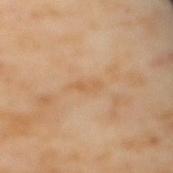notes: no biopsy performed (imaged during a skin exam) | lesion diameter: ~3 mm (longest diameter) | automated lesion analysis: a footprint of about 2.5 mm² and an eccentricity of roughly 0.95; a mean CIELAB color near L≈61 a*≈19 b*≈39, a lesion–skin lightness drop of about 6, and a normalized lesion–skin contrast near 5; a classifier nevus-likeness of about 0/100 and a detector confidence of about 100 out of 100 that the crop contains a lesion | anatomic site: the back | lighting: cross-polarized | subject: female, in their mid- to late 50s | image source: total-body-photography crop, ~15 mm field of view.